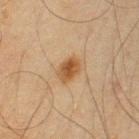<record>
  <biopsy_status>not biopsied; imaged during a skin examination</biopsy_status>
  <site>left upper arm</site>
  <patient>
    <sex>male</sex>
    <age_approx>65</age_approx>
  </patient>
  <lesion_size>
    <long_diameter_mm_approx>3.0</long_diameter_mm_approx>
  </lesion_size>
  <lighting>cross-polarized</lighting>
  <image>
    <source>total-body photography crop</source>
    <field_of_view_mm>15</field_of_view_mm>
  </image>
</record>